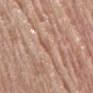Part of a total-body skin-imaging series; this lesion was reviewed on a skin check and was not flagged for biopsy. On the mid back. The lesion's longest dimension is about 2.5 mm. A region of skin cropped from a whole-body photographic capture, roughly 15 mm wide. Automated tile analysis of the lesion measured an average lesion color of about L≈57 a*≈20 b*≈29 (CIELAB), a lesion–skin lightness drop of about 7, and a lesion-to-skin contrast of about 5 (normalized; higher = more distinct). Imaged with white-light lighting. A female subject aged 73 to 77.